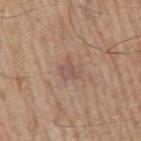Acquisition and patient details:
Longest diameter approximately 3 mm. Captured under white-light illumination. The subject is a male in their mid-70s. The lesion is on the right upper arm. An algorithmic analysis of the crop reported a footprint of about 5 mm². The software also gave a border-irregularity rating of about 3.5/10, internal color variation of about 4.5 on a 0–10 scale, and radial color variation of about 2. This image is a 15 mm lesion crop taken from a total-body photograph.